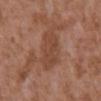The lesion was photographed on a routine skin check and not biopsied; there is no pathology result.
The recorded lesion diameter is about 5 mm.
This is a white-light tile.
A close-up tile cropped from a whole-body skin photograph, about 15 mm across.
A male subject roughly 75 years of age.
The lesion is on the abdomen.